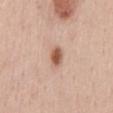notes: total-body-photography surveillance lesion; no biopsy
subject: female, about 45 years old
body site: the abdomen
imaging modality: ~15 mm crop, total-body skin-cancer survey